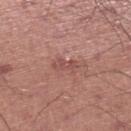{"biopsy_status": "not biopsied; imaged during a skin examination", "site": "right lower leg", "lesion_size": {"long_diameter_mm_approx": 2.5}, "automated_metrics": {"border_irregularity_0_10": 5.0, "color_variation_0_10": 0.0, "peripheral_color_asymmetry": 0.0}, "lighting": "white-light", "patient": {"sex": "male", "age_approx": 45}, "image": {"source": "total-body photography crop", "field_of_view_mm": 15}}